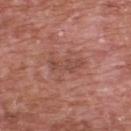Findings:
- workup — imaged on a skin check; not biopsied
- automated metrics — an average lesion color of about L≈48 a*≈24 b*≈26 (CIELAB), roughly 7 lightness units darker than nearby skin, and a normalized border contrast of about 5.5; a border-irregularity index near 5.5/10, a within-lesion color-variation index near 0.5/10, and peripheral color asymmetry of about 0; a lesion-detection confidence of about 100/100
- patient — male, approximately 70 years of age
- imaging modality — ~15 mm crop, total-body skin-cancer survey
- lesion size — ≈4 mm
- lighting — white-light illumination
- body site — the upper back